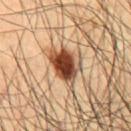Clinical impression: The lesion was tiled from a total-body skin photograph and was not biopsied. Context: The lesion-visualizer software estimated a border-irregularity rating of about 3.5/10, a color-variation rating of about 7/10, and radial color variation of about 2. A male subject, aged 48–52. This image is a 15 mm lesion crop taken from a total-body photograph. The lesion is on the chest. Imaged with cross-polarized lighting. Measured at roughly 5 mm in maximum diameter.